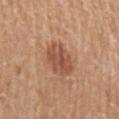Impression: Part of a total-body skin-imaging series; this lesion was reviewed on a skin check and was not flagged for biopsy. Background: From the arm. The recorded lesion diameter is about 3.5 mm. The patient is a female aged 73 to 77. Cropped from a whole-body photographic skin survey; the tile spans about 15 mm. The tile uses white-light illumination.